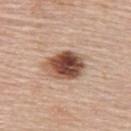Impression: Recorded during total-body skin imaging; not selected for excision or biopsy. Context: Captured under white-light illumination. A 15 mm crop from a total-body photograph taken for skin-cancer surveillance. An algorithmic analysis of the crop reported an area of roughly 13 mm², an outline eccentricity of about 0.7 (0 = round, 1 = elongated), and two-axis asymmetry of about 0.15. Located on the upper back. The patient is a female about 65 years old.